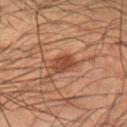Imaged during a routine full-body skin examination; the lesion was not biopsied and no histopathology is available. A male patient aged 48 to 52. Cropped from a whole-body photographic skin survey; the tile spans about 15 mm. This is a cross-polarized tile. An algorithmic analysis of the crop reported a detector confidence of about 100 out of 100 that the crop contains a lesion. Measured at roughly 3 mm in maximum diameter. Located on the left forearm.